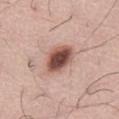The lesion was tiled from a total-body skin photograph and was not biopsied. The lesion is located on the abdomen. A 15 mm close-up extracted from a 3D total-body photography capture. The recorded lesion diameter is about 4.5 mm. The subject is a male aged approximately 60.